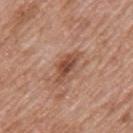<lesion>
<biopsy_status>not biopsied; imaged during a skin examination</biopsy_status>
<lighting>white-light</lighting>
<site>back</site>
<image>
  <source>total-body photography crop</source>
  <field_of_view_mm>15</field_of_view_mm>
</image>
<lesion_size>
  <long_diameter_mm_approx>4.0</long_diameter_mm_approx>
</lesion_size>
<automated_metrics>
  <area_mm2_approx>5.0</area_mm2_approx>
  <eccentricity>0.9</eccentricity>
  <cielab_L>48</cielab_L>
  <cielab_a>24</cielab_a>
  <cielab_b>30</cielab_b>
  <color_variation_0_10>3.0</color_variation_0_10>
</automated_metrics>
<patient>
  <sex>male</sex>
  <age_approx>60</age_approx>
</patient>
</lesion>